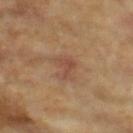Clinical impression:
The lesion was tiled from a total-body skin photograph and was not biopsied.